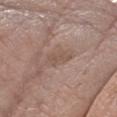The lesion was tiled from a total-body skin photograph and was not biopsied.
Imaged with white-light lighting.
The lesion is located on the right forearm.
A female subject, approximately 75 years of age.
Measured at roughly 3.5 mm in maximum diameter.
Cropped from a whole-body photographic skin survey; the tile spans about 15 mm.